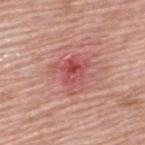  biopsy_status: not biopsied; imaged during a skin examination
  image:
    source: total-body photography crop
    field_of_view_mm: 15
  site: upper back
  patient:
    sex: male
    age_approx: 80
  lesion_size:
    long_diameter_mm_approx: 4.0
  automated_metrics:
    vs_skin_darker_L: 10.0
    vs_skin_contrast_norm: 6.5
    border_irregularity_0_10: 5.5
    color_variation_0_10: 7.0
    peripheral_color_asymmetry: 2.0
    lesion_detection_confidence_0_100: 100
  lighting: white-light